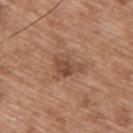Assessment:
No biopsy was performed on this lesion — it was imaged during a full skin examination and was not determined to be concerning.
Clinical summary:
A 15 mm crop from a total-body photograph taken for skin-cancer surveillance. The total-body-photography lesion software estimated a lesion area of about 8.5 mm², a shape eccentricity near 0.75, and two-axis asymmetry of about 0.45. It also reported an average lesion color of about L≈49 a*≈21 b*≈30 (CIELAB), roughly 9 lightness units darker than nearby skin, and a normalized lesion–skin contrast near 6.5. A male subject, in their 50s. The lesion is on the upper back. Measured at roughly 4.5 mm in maximum diameter.